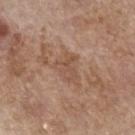biopsy status: total-body-photography surveillance lesion; no biopsy | imaging modality: 15 mm crop, total-body photography | image-analysis metrics: a lesion area of about 6 mm², an outline eccentricity of about 0.65 (0 = round, 1 = elongated), and a symmetry-axis asymmetry near 0.45; a border-irregularity index near 4.5/10, internal color variation of about 2.5 on a 0–10 scale, and peripheral color asymmetry of about 1 | lesion diameter: ~3.5 mm (longest diameter) | body site: the arm | patient: female, in their mid- to late 60s | tile lighting: white-light illumination.